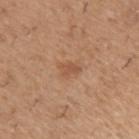| field | value |
|---|---|
| workup | total-body-photography surveillance lesion; no biopsy |
| location | the left upper arm |
| patient | male, aged approximately 50 |
| image source | 15 mm crop, total-body photography |
| lighting | white-light illumination |
| lesion diameter | about 2.5 mm |
| TBP lesion metrics | an automated nevus-likeness rating near 10 out of 100 |